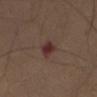Part of a total-body skin-imaging series; this lesion was reviewed on a skin check and was not flagged for biopsy.
The lesion is on the chest.
The lesion's longest dimension is about 2.5 mm.
Imaged with white-light lighting.
A male patient, aged approximately 75.
Cropped from a total-body skin-imaging series; the visible field is about 15 mm.
Automated image analysis of the tile measured a footprint of about 4.5 mm², a shape eccentricity near 0.65, and a symmetry-axis asymmetry near 0.35. And it measured an average lesion color of about L≈32 a*≈19 b*≈19 (CIELAB). The analysis additionally found a border-irregularity index near 3/10 and radial color variation of about 1.